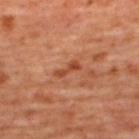– workup: no biopsy performed (imaged during a skin exam)
– patient: female, about 45 years old
– anatomic site: the upper back
– imaging modality: 15 mm crop, total-body photography
– lesion size: ~3 mm (longest diameter)
– automated lesion analysis: a footprint of about 3 mm² and a shape-asymmetry score of about 0.35 (0 = symmetric); a mean CIELAB color near L≈46 a*≈29 b*≈35 and a normalized lesion–skin contrast near 7
– lighting: cross-polarized illumination